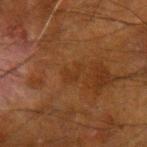Findings:
– follow-up — catalogued during a skin exam; not biopsied
– image — ~15 mm tile from a whole-body skin photo
– lesion size — about 2.5 mm
– lighting — cross-polarized
– site — the arm
– subject — male, approximately 65 years of age